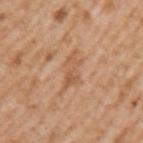Measured at roughly 5 mm in maximum diameter.
Located on the left upper arm.
Cropped from a whole-body photographic skin survey; the tile spans about 15 mm.
The patient is a male aged 63 to 67.